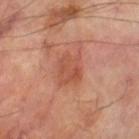This lesion was catalogued during total-body skin photography and was not selected for biopsy.
A male patient, in their 70s.
A region of skin cropped from a whole-body photographic capture, roughly 15 mm wide.
Longest diameter approximately 3.5 mm.
On the leg.
Captured under cross-polarized illumination.